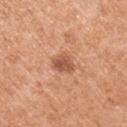No biopsy was performed on this lesion — it was imaged during a full skin examination and was not determined to be concerning. Approximately 3 mm at its widest. On the right upper arm. A lesion tile, about 15 mm wide, cut from a 3D total-body photograph. A male patient approximately 55 years of age. Imaged with white-light lighting. The total-body-photography lesion software estimated about 11 CIELAB-L* units darker than the surrounding skin. And it measured a border-irregularity index near 2/10, internal color variation of about 2.5 on a 0–10 scale, and radial color variation of about 1. The analysis additionally found a detector confidence of about 100 out of 100 that the crop contains a lesion.